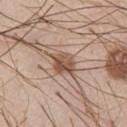Findings:
- notes — total-body-photography surveillance lesion; no biopsy
- automated lesion analysis — a lesion area of about 7 mm², an outline eccentricity of about 0.7 (0 = round, 1 = elongated), and two-axis asymmetry of about 0.2; a peripheral color-asymmetry measure near 2.5
- subject — male, in their 40s
- image — 15 mm crop, total-body photography
- lesion size — ~3.5 mm (longest diameter)
- site — the chest